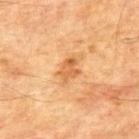Impression:
No biopsy was performed on this lesion — it was imaged during a full skin examination and was not determined to be concerning.
Image and clinical context:
A 15 mm close-up extracted from a 3D total-body photography capture. Captured under cross-polarized illumination. A male patient, roughly 75 years of age. The lesion is on the mid back.